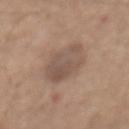<record>
<image>
  <source>total-body photography crop</source>
  <field_of_view_mm>15</field_of_view_mm>
</image>
<patient>
  <sex>male</sex>
  <age_approx>75</age_approx>
</patient>
<lighting>white-light</lighting>
<site>chest</site>
<lesion_size>
  <long_diameter_mm_approx>5.0</long_diameter_mm_approx>
</lesion_size>
</record>